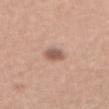workup: total-body-photography surveillance lesion; no biopsy | lesion size: about 3 mm | image source: 15 mm crop, total-body photography | patient: male, about 45 years old | body site: the mid back | tile lighting: white-light.